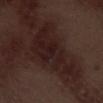Imaged during a routine full-body skin examination; the lesion was not biopsied and no histopathology is available.
From the leg.
The subject is a male about 70 years old.
Captured under white-light illumination.
A lesion tile, about 15 mm wide, cut from a 3D total-body photograph.